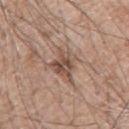Q: Is there a histopathology result?
A: no biopsy performed (imaged during a skin exam)
Q: Patient demographics?
A: male, approximately 60 years of age
Q: What kind of image is this?
A: 15 mm crop, total-body photography
Q: Automated lesion metrics?
A: an average lesion color of about L≈51 a*≈17 b*≈26 (CIELAB), about 11 CIELAB-L* units darker than the surrounding skin, and a normalized border contrast of about 7.5; a detector confidence of about 100 out of 100 that the crop contains a lesion
Q: Illumination type?
A: white-light illumination
Q: Where on the body is the lesion?
A: the arm
Q: What is the lesion's diameter?
A: about 4 mm